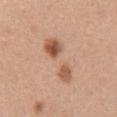tile lighting: white-light
site: the front of the torso
acquisition: ~15 mm tile from a whole-body skin photo
lesion diameter: ≈6 mm
subject: female, about 30 years old